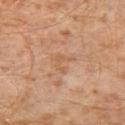Captured during whole-body skin photography for melanoma surveillance; the lesion was not biopsied.
The subject is a male approximately 30 years of age.
The lesion-visualizer software estimated an area of roughly 3.5 mm² and two-axis asymmetry of about 0.65. And it measured a nevus-likeness score of about 0/100 and a detector confidence of about 100 out of 100 that the crop contains a lesion.
The tile uses cross-polarized illumination.
A close-up tile cropped from a whole-body skin photograph, about 15 mm across.
On the left thigh.